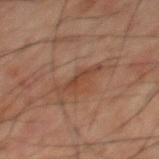workup = imaged on a skin check; not biopsied
acquisition = ~15 mm tile from a whole-body skin photo
anatomic site = the mid back
patient = male, roughly 60 years of age